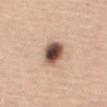Part of a total-body skin-imaging series; this lesion was reviewed on a skin check and was not flagged for biopsy.
Automated image analysis of the tile measured a footprint of about 8.5 mm². The software also gave a border-irregularity rating of about 1/10 and radial color variation of about 2.5. It also reported a nevus-likeness score of about 95/100 and lesion-presence confidence of about 100/100.
Imaged with white-light lighting.
Longest diameter approximately 3.5 mm.
A lesion tile, about 15 mm wide, cut from a 3D total-body photograph.
A female patient aged 53–57.
The lesion is on the abdomen.